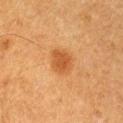follow-up — imaged on a skin check; not biopsied | body site — the right upper arm | patient — male, aged around 60 | image — ~15 mm tile from a whole-body skin photo.